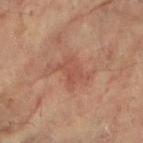The lesion was photographed on a routine skin check and not biopsied; there is no pathology result. On the arm. Captured under cross-polarized illumination. About 3.5 mm across. A female patient in their 80s. A lesion tile, about 15 mm wide, cut from a 3D total-body photograph.